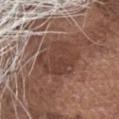The lesion was photographed on a routine skin check and not biopsied; there is no pathology result.
From the head or neck.
Captured under white-light illumination.
The lesion's longest dimension is about 9.5 mm.
A 15 mm crop from a total-body photograph taken for skin-cancer surveillance.
A male patient approximately 75 years of age.